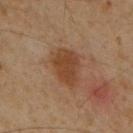• follow-up: imaged on a skin check; not biopsied
• site: the mid back
• image: ~15 mm tile from a whole-body skin photo
• illumination: cross-polarized
• automated metrics: an outline eccentricity of about 0.8 (0 = round, 1 = elongated)
• subject: male, roughly 60 years of age
• lesion size: about 5 mm